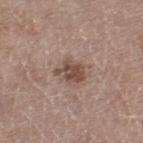Automated image analysis of the tile measured a lesion color around L≈47 a*≈18 b*≈24 in CIELAB, roughly 12 lightness units darker than nearby skin, and a normalized border contrast of about 9. It also reported a border-irregularity index near 4/10. This is a white-light tile. A close-up tile cropped from a whole-body skin photograph, about 15 mm across. Measured at roughly 3.5 mm in maximum diameter. A male subject aged approximately 65. The lesion is located on the left thigh.